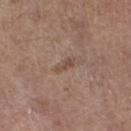Clinical impression: The lesion was tiled from a total-body skin photograph and was not biopsied. Acquisition and patient details: The recorded lesion diameter is about 2.5 mm. Located on the right lower leg. A female patient, in their mid-60s. A roughly 15 mm field-of-view crop from a total-body skin photograph. Imaged with white-light lighting.